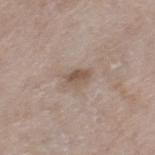Q: What is the lesion's diameter?
A: ~3 mm (longest diameter)
Q: Patient demographics?
A: female, aged approximately 75
Q: How was the tile lit?
A: white-light
Q: What kind of image is this?
A: ~15 mm crop, total-body skin-cancer survey
Q: Lesion location?
A: the left thigh